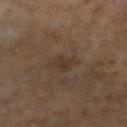Imaged during a routine full-body skin examination; the lesion was not biopsied and no histopathology is available.
Imaged with cross-polarized lighting.
A roughly 15 mm field-of-view crop from a total-body skin photograph.
About 3.5 mm across.
The lesion-visualizer software estimated an area of roughly 5 mm².
On the left leg.
The patient is a female in their mid- to late 60s.